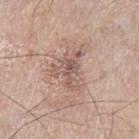{
  "biopsy_status": "not biopsied; imaged during a skin examination",
  "patient": {
    "sex": "male",
    "age_approx": 70
  },
  "lesion_size": {
    "long_diameter_mm_approx": 5.0
  },
  "image": {
    "source": "total-body photography crop",
    "field_of_view_mm": 15
  },
  "site": "left thigh",
  "lighting": "white-light"
}